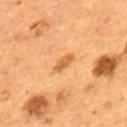Part of a total-body skin-imaging series; this lesion was reviewed on a skin check and was not flagged for biopsy.
Longest diameter approximately 3 mm.
Located on the upper back.
This image is a 15 mm lesion crop taken from a total-body photograph.
A male subject in their mid-50s.
This is a cross-polarized tile.